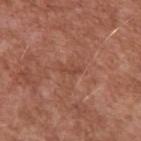biopsy status: total-body-photography surveillance lesion; no biopsy | automated lesion analysis: a footprint of about 2.5 mm², a shape eccentricity near 0.9, and two-axis asymmetry of about 0.4; an average lesion color of about L≈46 a*≈24 b*≈30 (CIELAB); a border-irregularity index near 4.5/10, internal color variation of about 0 on a 0–10 scale, and a peripheral color-asymmetry measure near 0 | illumination: white-light illumination | lesion size: ~2.5 mm (longest diameter) | patient: male, in their mid- to late 50s | acquisition: 15 mm crop, total-body photography | location: the left upper arm.